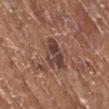Part of a total-body skin-imaging series; this lesion was reviewed on a skin check and was not flagged for biopsy.
A female patient aged 73 to 77.
A lesion tile, about 15 mm wide, cut from a 3D total-body photograph.
The lesion's longest dimension is about 4.5 mm.
Located on the right lower leg.